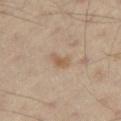The lesion was photographed on a routine skin check and not biopsied; there is no pathology result. A male patient, approximately 55 years of age. Cropped from a total-body skin-imaging series; the visible field is about 15 mm. The lesion is on the right thigh. The total-body-photography lesion software estimated a lesion area of about 3 mm² and two-axis asymmetry of about 0.4. The software also gave a mean CIELAB color near L≈57 a*≈16 b*≈32 and roughly 8 lightness units darker than nearby skin.